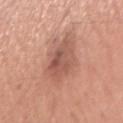Notes:
– workup: imaged on a skin check; not biopsied
– patient: male, in their 30s
– lesion size: about 5.5 mm
– lighting: white-light illumination
– acquisition: total-body-photography crop, ~15 mm field of view
– body site: the right upper arm